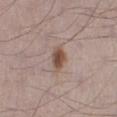follow-up: imaged on a skin check; not biopsied
imaging modality: total-body-photography crop, ~15 mm field of view
tile lighting: white-light illumination
lesion size: about 3 mm
subject: male, aged around 70
body site: the leg